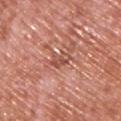Recorded during total-body skin imaging; not selected for excision or biopsy.
A male patient about 70 years old.
On the upper back.
The lesion's longest dimension is about 3 mm.
A 15 mm crop from a total-body photograph taken for skin-cancer surveillance.
Captured under white-light illumination.